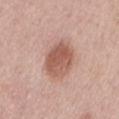Captured during whole-body skin photography for melanoma surveillance; the lesion was not biopsied. This image is a 15 mm lesion crop taken from a total-body photograph. The lesion is located on the mid back. Captured under white-light illumination. A male patient in their 70s.